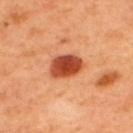No biopsy was performed on this lesion — it was imaged during a full skin examination and was not determined to be concerning.
The lesion is on the upper back.
A male patient approximately 50 years of age.
This is a cross-polarized tile.
A close-up tile cropped from a whole-body skin photograph, about 15 mm across.